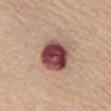No biopsy was performed on this lesion — it was imaged during a full skin examination and was not determined to be concerning.